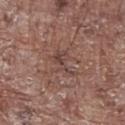Notes:
– biopsy status: total-body-photography surveillance lesion; no biopsy
– patient: male, approximately 70 years of age
– tile lighting: white-light illumination
– image source: ~15 mm crop, total-body skin-cancer survey
– anatomic site: the abdomen
– TBP lesion metrics: an outline eccentricity of about 0.85 (0 = round, 1 = elongated); an automated nevus-likeness rating near 0 out of 100 and lesion-presence confidence of about 65/100
– diameter: ≈4 mm